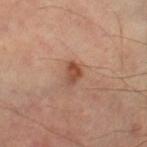Case summary:
- notes · total-body-photography surveillance lesion; no biopsy